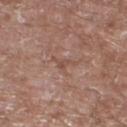– notes · imaged on a skin check; not biopsied
– acquisition · total-body-photography crop, ~15 mm field of view
– patient · male, aged around 60
– site · the right lower leg